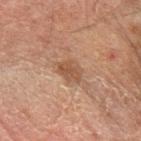Q: How was this image acquired?
A: total-body-photography crop, ~15 mm field of view
Q: Where on the body is the lesion?
A: the left forearm
Q: What did automated image analysis measure?
A: a lesion area of about 5 mm², an outline eccentricity of about 0.6 (0 = round, 1 = elongated), and a shape-asymmetry score of about 0.25 (0 = symmetric); border irregularity of about 2.5 on a 0–10 scale, a color-variation rating of about 2/10, and radial color variation of about 0.5
Q: Who is the patient?
A: male, aged approximately 65